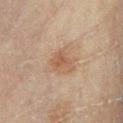The lesion was photographed on a routine skin check and not biopsied; there is no pathology result. On the left lower leg. Cropped from a total-body skin-imaging series; the visible field is about 15 mm. The subject is a male aged around 45.